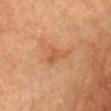• biopsy status · imaged on a skin check; not biopsied
• subject · female, about 65 years old
• site · the head or neck
• image · ~15 mm tile from a whole-body skin photo